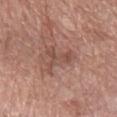workup: no biopsy performed (imaged during a skin exam)
illumination: white-light
diameter: about 4.5 mm
image-analysis metrics: a lesion color around L≈49 a*≈21 b*≈25 in CIELAB, roughly 8 lightness units darker than nearby skin, and a normalized lesion–skin contrast near 5.5
site: the right forearm
subject: female, aged around 60
image: 15 mm crop, total-body photography